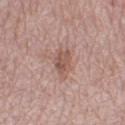biopsy_status: not biopsied; imaged during a skin examination
patient:
  sex: female
  age_approx: 40
image:
  source: total-body photography crop
  field_of_view_mm: 15
lighting: white-light
site: right thigh
lesion_size:
  long_diameter_mm_approx: 4.0